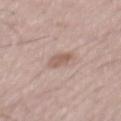Case summary:
* follow-up · imaged on a skin check; not biopsied
* acquisition · ~15 mm tile from a whole-body skin photo
* illumination · white-light illumination
* subject · male, in their mid- to late 50s
* lesion size · about 3 mm